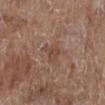Part of a total-body skin-imaging series; this lesion was reviewed on a skin check and was not flagged for biopsy.
A female subject, aged approximately 80.
Located on the right lower leg.
The tile uses white-light illumination.
A lesion tile, about 15 mm wide, cut from a 3D total-body photograph.
Measured at roughly 3 mm in maximum diameter.